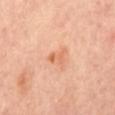Impression: The lesion was tiled from a total-body skin photograph and was not biopsied. Clinical summary: The lesion's longest dimension is about 3 mm. On the mid back. This is a cross-polarized tile. Automated tile analysis of the lesion measured a shape-asymmetry score of about 0.5 (0 = symmetric). The analysis additionally found a lesion color around L≈68 a*≈27 b*≈38 in CIELAB and about 9 CIELAB-L* units darker than the surrounding skin. And it measured an automated nevus-likeness rating near 25 out of 100 and lesion-presence confidence of about 100/100. A close-up tile cropped from a whole-body skin photograph, about 15 mm across.